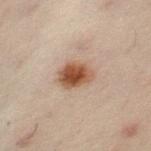location: the left lower leg
illumination: cross-polarized
image source: 15 mm crop, total-body photography
subject: female, aged 48–52
image-analysis metrics: an area of roughly 9 mm² and an outline eccentricity of about 0.5 (0 = round, 1 = elongated); a normalized border contrast of about 10.5; a within-lesion color-variation index near 5.5/10
size: ~3.5 mm (longest diameter)